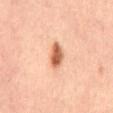Notes:
• lighting — cross-polarized illumination
• anatomic site — the back
• automated lesion analysis — a shape eccentricity near 0.85; border irregularity of about 2.5 on a 0–10 scale, a color-variation rating of about 4.5/10, and a peripheral color-asymmetry measure near 1.5
• subject — male, aged 58–62
• size — about 3.5 mm
• imaging modality — 15 mm crop, total-body photography